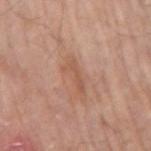Clinical impression:
Imaged during a routine full-body skin examination; the lesion was not biopsied and no histopathology is available.
Context:
Measured at roughly 3.5 mm in maximum diameter. A male patient roughly 70 years of age. A 15 mm close-up extracted from a 3D total-body photography capture. Captured under white-light illumination. The lesion is located on the right upper arm.